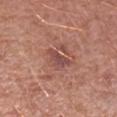notes: total-body-photography surveillance lesion; no biopsy | size: ~3 mm (longest diameter) | illumination: white-light illumination | site: the right forearm | image: total-body-photography crop, ~15 mm field of view | patient: female, roughly 40 years of age.